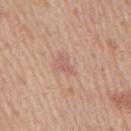Notes:
* workup: imaged on a skin check; not biopsied
* site: the right upper arm
* subject: male, in their mid-70s
* diameter: about 3 mm
* image-analysis metrics: a lesion color around L≈59 a*≈23 b*≈25 in CIELAB, a lesion–skin lightness drop of about 7, and a normalized border contrast of about 5; a border-irregularity rating of about 4/10, a within-lesion color-variation index near 0/10, and radial color variation of about 0; lesion-presence confidence of about 100/100
* imaging modality: ~15 mm crop, total-body skin-cancer survey
* lighting: white-light illumination